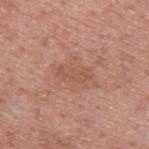workup=imaged on a skin check; not biopsied
image source=15 mm crop, total-body photography
illumination=white-light illumination
lesion diameter=~3.5 mm (longest diameter)
patient=male, approximately 55 years of age
body site=the upper back